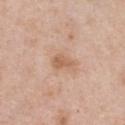Clinical impression:
The lesion was photographed on a routine skin check and not biopsied; there is no pathology result.
Background:
A female subject, aged approximately 55. Approximately 2.5 mm at its widest. From the chest. This image is a 15 mm lesion crop taken from a total-body photograph.